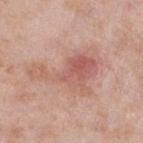The lesion was tiled from a total-body skin photograph and was not biopsied. The lesion's longest dimension is about 8 mm. A roughly 15 mm field-of-view crop from a total-body skin photograph. Located on the leg. This is a white-light tile. The total-body-photography lesion software estimated a border-irregularity index near 9/10, a within-lesion color-variation index near 5.5/10, and peripheral color asymmetry of about 1.5. And it measured a nevus-likeness score of about 5/100 and a lesion-detection confidence of about 100/100. A female subject aged around 60.